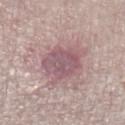The subject is a female roughly 70 years of age.
From the left lower leg.
A close-up tile cropped from a whole-body skin photograph, about 15 mm across.
The tile uses white-light illumination.
Measured at roughly 4.5 mm in maximum diameter.